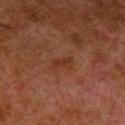No biopsy was performed on this lesion — it was imaged during a full skin examination and was not determined to be concerning. A close-up tile cropped from a whole-body skin photograph, about 15 mm across. The patient is a male approximately 80 years of age. The lesion is on the left lower leg.